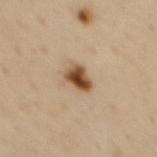image = 15 mm crop, total-body photography; site = the mid back; subject = male, aged approximately 55.